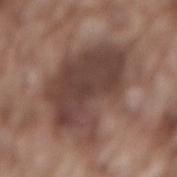workup: catalogued during a skin exam; not biopsied.